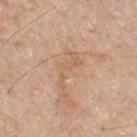biopsy status: imaged on a skin check; not biopsied.